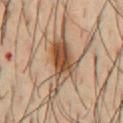{"image": {"source": "total-body photography crop", "field_of_view_mm": 15}, "automated_metrics": {"eccentricity": 0.9}, "patient": {"sex": "male", "age_approx": 40}, "site": "chest", "lighting": "cross-polarized"}